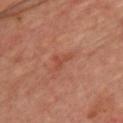The lesion was photographed on a routine skin check and not biopsied; there is no pathology result.
Imaged with cross-polarized lighting.
A subject aged around 55.
The recorded lesion diameter is about 2.5 mm.
From the chest.
A 15 mm close-up tile from a total-body photography series done for melanoma screening.